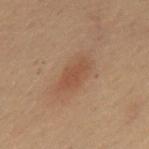Assessment: This lesion was catalogued during total-body skin photography and was not selected for biopsy. Image and clinical context: A male subject, aged around 55. On the mid back. A roughly 15 mm field-of-view crop from a total-body skin photograph.